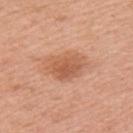TBP lesion metrics: a footprint of about 12 mm², an outline eccentricity of about 0.7 (0 = round, 1 = elongated), and a symmetry-axis asymmetry near 0.2; a border-irregularity index near 2.5/10 and a color-variation rating of about 3.5/10; an automated nevus-likeness rating near 40 out of 100 and lesion-presence confidence of about 100/100 | subject: female, aged approximately 50 | lighting: white-light illumination | body site: the left upper arm | image: ~15 mm tile from a whole-body skin photo.